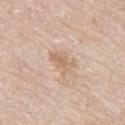automated metrics: internal color variation of about 2 on a 0–10 scale and a peripheral color-asymmetry measure near 0.5 | patient: male, aged around 80 | image: ~15 mm tile from a whole-body skin photo | anatomic site: the left thigh.